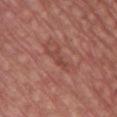Clinical impression: The lesion was photographed on a routine skin check and not biopsied; there is no pathology result. Image and clinical context: Automated image analysis of the tile measured an area of roughly 3.5 mm², an outline eccentricity of about 0.9 (0 = round, 1 = elongated), and two-axis asymmetry of about 0.45. It also reported a border-irregularity index near 6/10 and radial color variation of about 0. It also reported a nevus-likeness score of about 0/100. A male subject, approximately 60 years of age. The recorded lesion diameter is about 3.5 mm. A 15 mm crop from a total-body photograph taken for skin-cancer surveillance. The lesion is located on the chest.